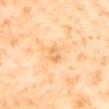Q: What did automated image analysis measure?
A: about 8 CIELAB-L* units darker than the surrounding skin
Q: What is the lesion's diameter?
A: ~2.5 mm (longest diameter)
Q: How was the tile lit?
A: cross-polarized illumination
Q: Lesion location?
A: the back
Q: Patient demographics?
A: female, aged 53–57
Q: What kind of image is this?
A: total-body-photography crop, ~15 mm field of view